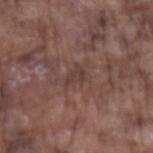The lesion is on the left forearm. Automated tile analysis of the lesion measured a lesion area of about 3 mm², an outline eccentricity of about 0.8 (0 = round, 1 = elongated), and two-axis asymmetry of about 0.4. The analysis additionally found a border-irregularity rating of about 4.5/10, a within-lesion color-variation index near 1/10, and radial color variation of about 0.5. The software also gave an automated nevus-likeness rating near 0 out of 100 and a detector confidence of about 75 out of 100 that the crop contains a lesion. A roughly 15 mm field-of-view crop from a total-body skin photograph. A male subject, aged around 75.